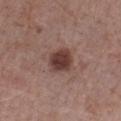Impression:
No biopsy was performed on this lesion — it was imaged during a full skin examination and was not determined to be concerning.
Acquisition and patient details:
A male subject in their mid- to late 70s. Longest diameter approximately 3.5 mm. A 15 mm crop from a total-body photograph taken for skin-cancer surveillance. The total-body-photography lesion software estimated a footprint of about 8 mm², an eccentricity of roughly 0.5, and a shape-asymmetry score of about 0.1 (0 = symmetric). It also reported roughly 13 lightness units darker than nearby skin and a normalized lesion–skin contrast near 10.5. The analysis additionally found a border-irregularity rating of about 1/10, internal color variation of about 3.5 on a 0–10 scale, and peripheral color asymmetry of about 1. And it measured an automated nevus-likeness rating near 95 out of 100 and lesion-presence confidence of about 100/100. From the left forearm.